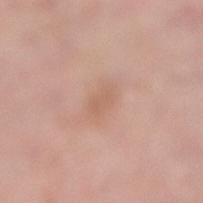workup: imaged on a skin check; not biopsied
site: the right lower leg
subject: female, in their mid-50s
image: ~15 mm tile from a whole-body skin photo
lighting: white-light